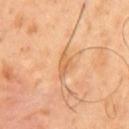Clinical impression:
Imaged during a routine full-body skin examination; the lesion was not biopsied and no histopathology is available.
Image and clinical context:
The tile uses cross-polarized illumination. The recorded lesion diameter is about 3 mm. Located on the front of the torso. Automated tile analysis of the lesion measured an average lesion color of about L≈64 a*≈23 b*≈41 (CIELAB) and a normalized border contrast of about 6. A roughly 15 mm field-of-view crop from a total-body skin photograph. A male patient roughly 50 years of age.